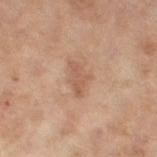Image and clinical context: Approximately 3.5 mm at its widest. The total-body-photography lesion software estimated a lesion color around L≈48 a*≈18 b*≈27 in CIELAB, a lesion–skin lightness drop of about 7, and a lesion-to-skin contrast of about 5.5 (normalized; higher = more distinct). The software also gave border irregularity of about 4.5 on a 0–10 scale, internal color variation of about 0.5 on a 0–10 scale, and a peripheral color-asymmetry measure near 0. A 15 mm crop from a total-body photograph taken for skin-cancer surveillance. A female patient about 60 years old. The lesion is on the leg. Captured under cross-polarized illumination.